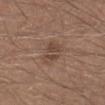The lesion was tiled from a total-body skin photograph and was not biopsied.
The recorded lesion diameter is about 3.5 mm.
The lesion is on the left lower leg.
The subject is a male approximately 30 years of age.
A 15 mm crop from a total-body photograph taken for skin-cancer surveillance.
This is a white-light tile.